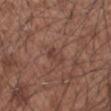Findings:
• lighting: white-light illumination
• patient: male, aged around 55
• automated metrics: a footprint of about 3 mm²; a mean CIELAB color near L≈40 a*≈20 b*≈24, roughly 7 lightness units darker than nearby skin, and a normalized border contrast of about 6; a border-irregularity index near 3.5/10, a color-variation rating of about 1/10, and radial color variation of about 0; a nevus-likeness score of about 0/100 and a lesion-detection confidence of about 100/100
• imaging modality: total-body-photography crop, ~15 mm field of view
• anatomic site: the arm
• lesion diameter: ≈2.5 mm